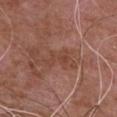The lesion was photographed on a routine skin check and not biopsied; there is no pathology result.
A lesion tile, about 15 mm wide, cut from a 3D total-body photograph.
The lesion's longest dimension is about 3.5 mm.
From the chest.
A male subject, roughly 75 years of age.
This is a white-light tile.